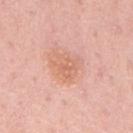Cropped from a total-body skin-imaging series; the visible field is about 15 mm. A male patient aged 63 to 67. From the mid back.